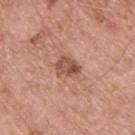Impression: Recorded during total-body skin imaging; not selected for excision or biopsy. Background: The subject is a male in their mid-50s. A close-up tile cropped from a whole-body skin photograph, about 15 mm across. The lesion is located on the upper back. Approximately 3 mm at its widest. The tile uses white-light illumination.